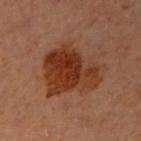Notes:
* notes · imaged on a skin check; not biopsied
* TBP lesion metrics · a footprint of about 27 mm² and an eccentricity of roughly 0.7
* anatomic site · the left arm
* size · ≈7 mm
* image source · ~15 mm crop, total-body skin-cancer survey
* subject · female, in their 60s